Cropped from a total-body skin-imaging series; the visible field is about 15 mm. On the upper back. A female subject approximately 70 years of age:
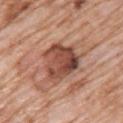The lesion was biopsied, and histopathology showed a melanoma in situ arising in association with a nevus.